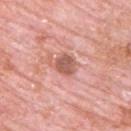The lesion was photographed on a routine skin check and not biopsied; there is no pathology result. Captured under white-light illumination. Longest diameter approximately 3 mm. A male subject aged 78–82. A 15 mm close-up extracted from a 3D total-body photography capture. Located on the upper back.